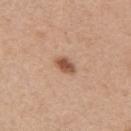Imaged during a routine full-body skin examination; the lesion was not biopsied and no histopathology is available.
A female patient approximately 45 years of age.
The lesion's longest dimension is about 2.5 mm.
Located on the right upper arm.
A 15 mm crop from a total-body photograph taken for skin-cancer surveillance.